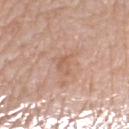Assessment:
The lesion was photographed on a routine skin check and not biopsied; there is no pathology result.
Background:
A female subject approximately 70 years of age. This is a white-light tile. The lesion is located on the leg. A 15 mm crop from a total-body photograph taken for skin-cancer surveillance.